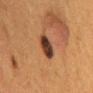notes = total-body-photography surveillance lesion; no biopsy
location = the back
acquisition = ~15 mm crop, total-body skin-cancer survey
illumination = cross-polarized illumination
patient = female, in their 50s